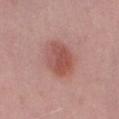This lesion was catalogued during total-body skin photography and was not selected for biopsy.
The lesion is on the left thigh.
The lesion-visualizer software estimated a lesion area of about 14 mm² and a symmetry-axis asymmetry near 0.1. The analysis additionally found a lesion color around L≈53 a*≈26 b*≈25 in CIELAB and roughly 10 lightness units darker than nearby skin. And it measured a nevus-likeness score of about 100/100 and a lesion-detection confidence of about 100/100.
A male subject roughly 40 years of age.
The lesion's longest dimension is about 5 mm.
A region of skin cropped from a whole-body photographic capture, roughly 15 mm wide.
This is a white-light tile.